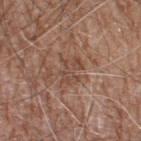The lesion was photographed on a routine skin check and not biopsied; there is no pathology result. A male patient roughly 60 years of age. From the right thigh. The recorded lesion diameter is about 3 mm. Automated tile analysis of the lesion measured a normalized lesion–skin contrast near 5.5. It also reported a color-variation rating of about 1.5/10 and radial color variation of about 0.5. A 15 mm close-up extracted from a 3D total-body photography capture.